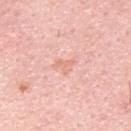Impression:
Captured during whole-body skin photography for melanoma surveillance; the lesion was not biopsied.
Context:
A male patient, approximately 30 years of age. This is a white-light tile. Automated tile analysis of the lesion measured a footprint of about 3 mm², an eccentricity of roughly 0.7, and two-axis asymmetry of about 0.45. And it measured a detector confidence of about 100 out of 100 that the crop contains a lesion. Located on the back. A roughly 15 mm field-of-view crop from a total-body skin photograph. Approximately 2.5 mm at its widest.